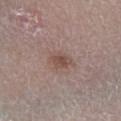illumination: white-light illumination | subject: female, roughly 65 years of age | lesion diameter: ~3 mm (longest diameter) | image-analysis metrics: a lesion area of about 5 mm² and two-axis asymmetry of about 0.25; internal color variation of about 3 on a 0–10 scale and peripheral color asymmetry of about 1 | imaging modality: ~15 mm tile from a whole-body skin photo | location: the right lower leg.